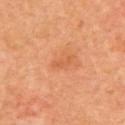Case summary:
– workup — no biopsy performed (imaged during a skin exam)
– automated metrics — an area of roughly 2 mm², an outline eccentricity of about 0.9 (0 = round, 1 = elongated), and two-axis asymmetry of about 0.5; a lesion–skin lightness drop of about 7 and a normalized border contrast of about 5; peripheral color asymmetry of about 0; an automated nevus-likeness rating near 0 out of 100 and a detector confidence of about 100 out of 100 that the crop contains a lesion
– subject — male, aged around 65
– imaging modality — 15 mm crop, total-body photography
– location — the upper back